On the right upper arm; a male patient in their 60s; a close-up tile cropped from a whole-body skin photograph, about 15 mm across:
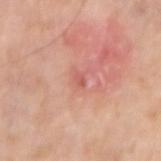Image and clinical context:
The lesion's longest dimension is about 1.5 mm. Imaged with white-light lighting.
Diagnosis:
On excision, pathology confirmed a squamous cell carcinoma in situ, classified as a malignant lesion.